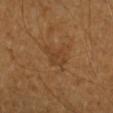Case summary:
– TBP lesion metrics · a footprint of about 5 mm² and an outline eccentricity of about 0.75 (0 = round, 1 = elongated); border irregularity of about 5 on a 0–10 scale, a within-lesion color-variation index near 1.5/10, and peripheral color asymmetry of about 0.5
– image · ~15 mm tile from a whole-body skin photo
– size · about 3.5 mm
– illumination · cross-polarized illumination
– body site · the right forearm
– patient · male, aged around 55